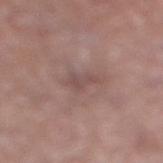Impression:
Imaged during a routine full-body skin examination; the lesion was not biopsied and no histopathology is available.
Acquisition and patient details:
The tile uses white-light illumination. The recorded lesion diameter is about 2.5 mm. Located on the right lower leg. Cropped from a whole-body photographic skin survey; the tile spans about 15 mm. A male patient aged approximately 70.